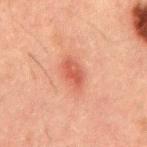The lesion was photographed on a routine skin check and not biopsied; there is no pathology result. A male patient, about 50 years old. The tile uses cross-polarized illumination. A roughly 15 mm field-of-view crop from a total-body skin photograph. On the back. Measured at roughly 3.5 mm in maximum diameter.